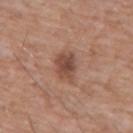Impression:
Part of a total-body skin-imaging series; this lesion was reviewed on a skin check and was not flagged for biopsy.
Image and clinical context:
A male subject, aged 58–62. The total-body-photography lesion software estimated a lesion area of about 7 mm², a shape eccentricity near 0.75, and a shape-asymmetry score of about 0.25 (0 = symmetric). It also reported a lesion color around L≈47 a*≈21 b*≈27 in CIELAB, roughly 11 lightness units darker than nearby skin, and a lesion-to-skin contrast of about 8 (normalized; higher = more distinct). The software also gave a border-irregularity index near 2.5/10 and a within-lesion color-variation index near 3/10. It also reported a detector confidence of about 100 out of 100 that the crop contains a lesion. The lesion is on the front of the torso. Measured at roughly 4 mm in maximum diameter. A 15 mm close-up extracted from a 3D total-body photography capture.